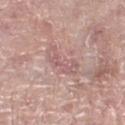diameter: ≈4.5 mm
automated metrics: a lesion area of about 7 mm², a shape eccentricity near 0.9, and a shape-asymmetry score of about 0.4 (0 = symmetric); a mean CIELAB color near L≈59 a*≈21 b*≈20, roughly 7 lightness units darker than nearby skin, and a normalized border contrast of about 5
image: ~15 mm crop, total-body skin-cancer survey
subject: female, aged approximately 60
illumination: white-light
anatomic site: the left lower leg